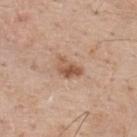Clinical impression:
The lesion was tiled from a total-body skin photograph and was not biopsied.
Acquisition and patient details:
The total-body-photography lesion software estimated a footprint of about 4.5 mm², a shape eccentricity near 0.75, and two-axis asymmetry of about 0.3. And it measured a lesion-detection confidence of about 100/100. About 3 mm across. Cropped from a total-body skin-imaging series; the visible field is about 15 mm. Imaged with white-light lighting. The subject is a male aged 58 to 62. From the upper back.